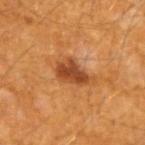biopsy status: total-body-photography surveillance lesion; no biopsy | body site: the left forearm | imaging modality: 15 mm crop, total-body photography | tile lighting: cross-polarized | lesion diameter: ≈4.5 mm | image-analysis metrics: an area of roughly 9 mm², a shape eccentricity near 0.85, and two-axis asymmetry of about 0.35; border irregularity of about 3.5 on a 0–10 scale, a color-variation rating of about 4/10, and a peripheral color-asymmetry measure near 1; lesion-presence confidence of about 100/100 | subject: male, roughly 60 years of age.